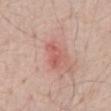biopsy_status: not biopsied; imaged during a skin examination
lighting: white-light
site: chest
lesion_size:
  long_diameter_mm_approx: 3.5
image:
  source: total-body photography crop
  field_of_view_mm: 15
patient:
  sex: male
  age_approx: 70